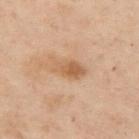Impression: Part of a total-body skin-imaging series; this lesion was reviewed on a skin check and was not flagged for biopsy. Image and clinical context: Imaged with cross-polarized lighting. A region of skin cropped from a whole-body photographic capture, roughly 15 mm wide. A female subject aged 53 to 57. From the chest. Approximately 4 mm at its widest.